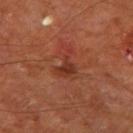A region of skin cropped from a whole-body photographic capture, roughly 15 mm wide. The patient is a male roughly 65 years of age. The lesion is located on the leg.